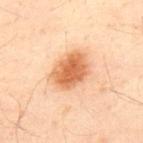Clinical impression: No biopsy was performed on this lesion — it was imaged during a full skin examination and was not determined to be concerning. Context: The lesion-visualizer software estimated a nevus-likeness score of about 100/100 and a lesion-detection confidence of about 100/100. A male subject aged 48 to 52. From the upper back. The recorded lesion diameter is about 5 mm. A close-up tile cropped from a whole-body skin photograph, about 15 mm across. Imaged with cross-polarized lighting.